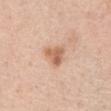Imaged with white-light lighting. A 15 mm close-up tile from a total-body photography series done for melanoma screening. The lesion's longest dimension is about 3 mm. A female patient about 55 years old. The lesion is located on the chest.